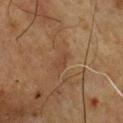Clinical impression: The lesion was photographed on a routine skin check and not biopsied; there is no pathology result. Background: Captured under cross-polarized illumination. A male patient in their 60s. A 15 mm close-up tile from a total-body photography series done for melanoma screening. From the front of the torso.